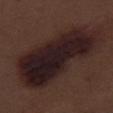{
  "image": {
    "source": "total-body photography crop",
    "field_of_view_mm": 15
  },
  "automated_metrics": {
    "cielab_L": 20,
    "cielab_a": 15,
    "cielab_b": 14,
    "vs_skin_darker_L": 11.0,
    "vs_skin_contrast_norm": 14.0
  },
  "lighting": "white-light",
  "patient": {
    "sex": "male",
    "age_approx": 70
  },
  "lesion_size": {
    "long_diameter_mm_approx": 13.5
  },
  "site": "right thigh"
}